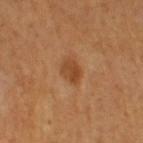{
  "biopsy_status": "not biopsied; imaged during a skin examination",
  "site": "leg",
  "image": {
    "source": "total-body photography crop",
    "field_of_view_mm": 15
  },
  "patient": {
    "sex": "female",
    "age_approx": 55
  },
  "lighting": "cross-polarized",
  "lesion_size": {
    "long_diameter_mm_approx": 2.5
  }
}